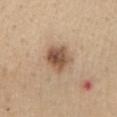Part of a total-body skin-imaging series; this lesion was reviewed on a skin check and was not flagged for biopsy. Automated image analysis of the tile measured about 14 CIELAB-L* units darker than the surrounding skin. The software also gave a border-irregularity rating of about 2/10, a color-variation rating of about 6/10, and peripheral color asymmetry of about 2. A female patient in their 60s. Approximately 3.5 mm at its widest. This image is a 15 mm lesion crop taken from a total-body photograph. On the chest. The tile uses white-light illumination.